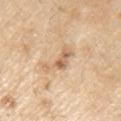follow-up — no biopsy performed (imaged during a skin exam)
patient — male, aged around 70
acquisition — ~15 mm crop, total-body skin-cancer survey
illumination — white-light illumination
automated lesion analysis — an outline eccentricity of about 0.9 (0 = round, 1 = elongated); a within-lesion color-variation index near 4.5/10 and a peripheral color-asymmetry measure near 1.5; a lesion-detection confidence of about 50/100
diameter — ≈4.5 mm
site — the left upper arm